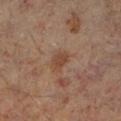A male subject, aged approximately 60.
A roughly 15 mm field-of-view crop from a total-body skin photograph.
On the left lower leg.